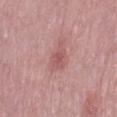{"biopsy_status": "not biopsied; imaged during a skin examination", "automated_metrics": {"area_mm2_approx": 6.5, "eccentricity": 0.9, "shape_asymmetry": 0.4, "cielab_L": 56, "cielab_a": 25, "cielab_b": 22, "vs_skin_darker_L": 8.0}, "lesion_size": {"long_diameter_mm_approx": 4.0}, "image": {"source": "total-body photography crop", "field_of_view_mm": 15}, "patient": {"sex": "female", "age_approx": 70}, "lighting": "white-light", "site": "right thigh"}